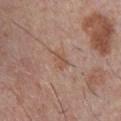{"site": "chest", "lesion_size": {"long_diameter_mm_approx": 2.5}, "image": {"source": "total-body photography crop", "field_of_view_mm": 15}, "lighting": "white-light", "patient": {"sex": "male", "age_approx": 30}}